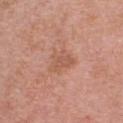Recorded during total-body skin imaging; not selected for excision or biopsy. From the chest. Cropped from a total-body skin-imaging series; the visible field is about 15 mm. About 3 mm across. A male patient in their 70s.